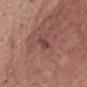The lesion was tiled from a total-body skin photograph and was not biopsied.
The recorded lesion diameter is about 2.5 mm.
This is a white-light tile.
A male patient, aged 78–82.
From the front of the torso.
Cropped from a total-body skin-imaging series; the visible field is about 15 mm.